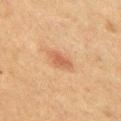biopsy status: total-body-photography surveillance lesion; no biopsy
image-analysis metrics: border irregularity of about 3 on a 0–10 scale, a color-variation rating of about 1.5/10, and peripheral color asymmetry of about 0.5
acquisition: ~15 mm tile from a whole-body skin photo
body site: the chest
lesion size: ~3 mm (longest diameter)
patient: male, aged 48 to 52